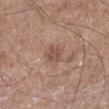notes = imaged on a skin check; not biopsied | automated lesion analysis = a footprint of about 4.5 mm² and a symmetry-axis asymmetry near 0.3; a border-irregularity index near 3/10, internal color variation of about 1.5 on a 0–10 scale, and peripheral color asymmetry of about 0.5; lesion-presence confidence of about 100/100 | size = about 3 mm | tile lighting = white-light illumination | body site = the right lower leg | image = ~15 mm crop, total-body skin-cancer survey | subject = male, aged 58–62.